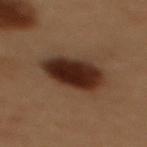| field | value |
|---|---|
| follow-up | imaged on a skin check; not biopsied |
| subject | female, aged around 50 |
| imaging modality | 15 mm crop, total-body photography |
| lighting | cross-polarized illumination |
| anatomic site | the upper back |
| diameter | ≈5.5 mm |